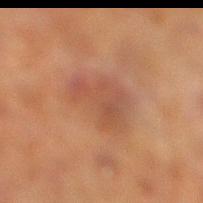This lesion was catalogued during total-body skin photography and was not selected for biopsy.
The lesion is on the right lower leg.
A roughly 15 mm field-of-view crop from a total-body skin photograph.
Captured under cross-polarized illumination.
Automated image analysis of the tile measured an outline eccentricity of about 0.7 (0 = round, 1 = elongated) and a symmetry-axis asymmetry near 0.25. The software also gave about 6 CIELAB-L* units darker than the surrounding skin and a normalized lesion–skin contrast near 5.
A male patient about 70 years old.
About 5.5 mm across.